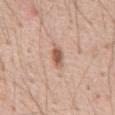Part of a total-body skin-imaging series; this lesion was reviewed on a skin check and was not flagged for biopsy. A 15 mm close-up tile from a total-body photography series done for melanoma screening. The lesion is located on the abdomen. The lesion-visualizer software estimated an average lesion color of about L≈57 a*≈21 b*≈31 (CIELAB), a lesion–skin lightness drop of about 13, and a normalized lesion–skin contrast near 8.5. And it measured a border-irregularity index near 1.5/10 and a within-lesion color-variation index near 3.5/10. The subject is a male about 55 years old.